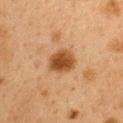Part of a total-body skin-imaging series; this lesion was reviewed on a skin check and was not flagged for biopsy. The lesion is located on the upper back. A female patient, aged approximately 40. The lesion's longest dimension is about 3.5 mm. Cropped from a whole-body photographic skin survey; the tile spans about 15 mm. Captured under cross-polarized illumination. The total-body-photography lesion software estimated a lesion-detection confidence of about 100/100.